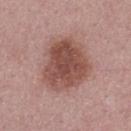Clinical impression:
Imaged during a routine full-body skin examination; the lesion was not biopsied and no histopathology is available.
Image and clinical context:
An algorithmic analysis of the crop reported a shape eccentricity near 0.45 and a shape-asymmetry score of about 0.2 (0 = symmetric). It also reported internal color variation of about 4.5 on a 0–10 scale and peripheral color asymmetry of about 1.5. A 15 mm crop from a total-body photograph taken for skin-cancer surveillance. The lesion's longest dimension is about 6.5 mm. This is a white-light tile. A male subject roughly 30 years of age.